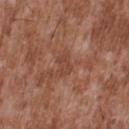Part of a total-body skin-imaging series; this lesion was reviewed on a skin check and was not flagged for biopsy. A male subject, in their mid-40s. A region of skin cropped from a whole-body photographic capture, roughly 15 mm wide. Measured at roughly 3 mm in maximum diameter. The lesion is on the upper back. The total-body-photography lesion software estimated a lesion area of about 4.5 mm², an eccentricity of roughly 0.75, and a symmetry-axis asymmetry near 0.25. The analysis additionally found a mean CIELAB color near L≈44 a*≈23 b*≈29. And it measured a border-irregularity rating of about 2.5/10, a color-variation rating of about 1.5/10, and peripheral color asymmetry of about 0.5. And it measured lesion-presence confidence of about 100/100.